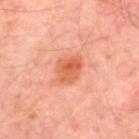Notes:
• workup · total-body-photography surveillance lesion; no biopsy
• size · about 4 mm
• tile lighting · cross-polarized
• image · total-body-photography crop, ~15 mm field of view
• subject · approximately 55 years of age
• site · the upper back